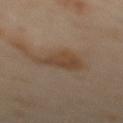Impression:
Captured during whole-body skin photography for melanoma surveillance; the lesion was not biopsied.
Background:
A 15 mm crop from a total-body photograph taken for skin-cancer surveillance. A male patient, aged 63 to 67. The lesion is located on the mid back. Imaged with cross-polarized lighting. Automated tile analysis of the lesion measured a footprint of about 10 mm², a shape eccentricity near 0.7, and a shape-asymmetry score of about 0.45 (0 = symmetric). The analysis additionally found a lesion color around L≈42 a*≈15 b*≈28 in CIELAB, roughly 7 lightness units darker than nearby skin, and a normalized border contrast of about 6.5. It also reported a border-irregularity index near 4.5/10, a within-lesion color-variation index near 2.5/10, and peripheral color asymmetry of about 1.